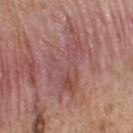Captured during whole-body skin photography for melanoma surveillance; the lesion was not biopsied.